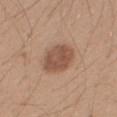<tbp_lesion>
  <biopsy_status>not biopsied; imaged during a skin examination</biopsy_status>
  <site>right forearm</site>
  <patient>
    <sex>male</sex>
    <age_approx>20</age_approx>
  </patient>
  <image>
    <source>total-body photography crop</source>
    <field_of_view_mm>15</field_of_view_mm>
  </image>
</tbp_lesion>